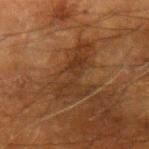Imaged during a routine full-body skin examination; the lesion was not biopsied and no histopathology is available. A male patient, approximately 65 years of age. From the left forearm. A close-up tile cropped from a whole-body skin photograph, about 15 mm across.